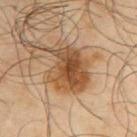{
  "biopsy_status": "not biopsied; imaged during a skin examination",
  "patient": {
    "sex": "male",
    "age_approx": 65
  },
  "image": {
    "source": "total-body photography crop",
    "field_of_view_mm": 15
  },
  "site": "right upper arm",
  "automated_metrics": {
    "area_mm2_approx": 20.0,
    "eccentricity": 0.65,
    "shape_asymmetry": 0.25,
    "cielab_L": 47,
    "cielab_a": 18,
    "cielab_b": 34,
    "vs_skin_contrast_norm": 9.5,
    "border_irregularity_0_10": 3.5,
    "color_variation_0_10": 6.5,
    "peripheral_color_asymmetry": 2.0
  }
}